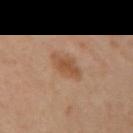<record>
  <biopsy_status>not biopsied; imaged during a skin examination</biopsy_status>
  <image>
    <source>total-body photography crop</source>
    <field_of_view_mm>15</field_of_view_mm>
  </image>
  <site>arm</site>
  <patient>
    <sex>female</sex>
    <age_approx>40</age_approx>
  </patient>
</record>